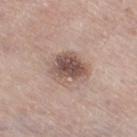- subject · male, aged approximately 85 or older
- image · total-body-photography crop, ~15 mm field of view
- diameter · ≈4.5 mm
- body site · the right thigh